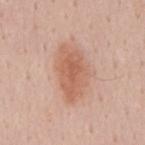Clinical impression: Imaged during a routine full-body skin examination; the lesion was not biopsied and no histopathology is available. Image and clinical context: The lesion is on the mid back. A male patient aged approximately 55. Imaged with white-light lighting. A roughly 15 mm field-of-view crop from a total-body skin photograph.